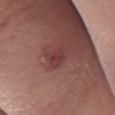notes: no biopsy performed (imaged during a skin exam) | lighting: white-light illumination | automated metrics: a footprint of about 3.5 mm², an eccentricity of roughly 0.75, and two-axis asymmetry of about 0.2; a lesion color around L≈38 a*≈26 b*≈20 in CIELAB and about 7 CIELAB-L* units darker than the surrounding skin; a classifier nevus-likeness of about 5/100 and lesion-presence confidence of about 100/100 | patient: male, aged around 55 | anatomic site: the right lower leg | diameter: ~2.5 mm (longest diameter) | image: ~15 mm tile from a whole-body skin photo.